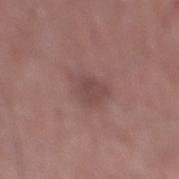The lesion was tiled from a total-body skin photograph and was not biopsied. The lesion is located on the right lower leg. A 15 mm close-up extracted from a 3D total-body photography capture. An algorithmic analysis of the crop reported a mean CIELAB color near L≈47 a*≈19 b*≈20 and a normalized lesion–skin contrast near 5.5. The software also gave a classifier nevus-likeness of about 0/100 and lesion-presence confidence of about 100/100. Imaged with white-light lighting. About 4 mm across. A male subject aged 58–62.